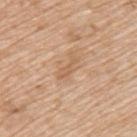Imaged during a routine full-body skin examination; the lesion was not biopsied and no histopathology is available.
Captured under white-light illumination.
A male subject, roughly 65 years of age.
A 15 mm close-up extracted from a 3D total-body photography capture.
Located on the back.